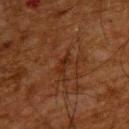biopsy status: no biopsy performed (imaged during a skin exam)
anatomic site: the upper back
image-analysis metrics: an average lesion color of about L≈23 a*≈19 b*≈26 (CIELAB), roughly 5 lightness units darker than nearby skin, and a normalized border contrast of about 6; border irregularity of about 3 on a 0–10 scale, a within-lesion color-variation index near 0/10, and radial color variation of about 0; an automated nevus-likeness rating near 0 out of 100 and a detector confidence of about 90 out of 100 that the crop contains a lesion
patient: male, roughly 65 years of age
tile lighting: cross-polarized
image source: ~15 mm crop, total-body skin-cancer survey
diameter: ≈2.5 mm